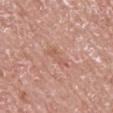Captured during whole-body skin photography for melanoma surveillance; the lesion was not biopsied. On the back. A lesion tile, about 15 mm wide, cut from a 3D total-body photograph. Measured at roughly 3 mm in maximum diameter. The total-body-photography lesion software estimated a lesion area of about 2.5 mm² and two-axis asymmetry of about 0.45. Imaged with white-light lighting. A male subject in their 70s.